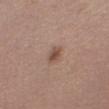{"site": "right thigh", "lesion_size": {"long_diameter_mm_approx": 2.5}, "lighting": "white-light", "image": {"source": "total-body photography crop", "field_of_view_mm": 15}, "patient": {"sex": "female", "age_approx": 50}}